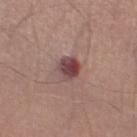Captured during whole-body skin photography for melanoma surveillance; the lesion was not biopsied.
The lesion is located on the left thigh.
The subject is a male aged around 55.
Automated tile analysis of the lesion measured a footprint of about 6.5 mm² and a shape eccentricity near 0.7. The analysis additionally found a border-irregularity rating of about 1.5/10, a within-lesion color-variation index near 7.5/10, and radial color variation of about 2.5. The analysis additionally found a classifier nevus-likeness of about 65/100.
This is a white-light tile.
A 15 mm crop from a total-body photograph taken for skin-cancer surveillance.
Measured at roughly 3.5 mm in maximum diameter.